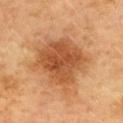Assessment:
This lesion was catalogued during total-body skin photography and was not selected for biopsy.
Acquisition and patient details:
Imaged with cross-polarized lighting. The lesion is located on the upper back. An algorithmic analysis of the crop reported an outline eccentricity of about 0.35 (0 = round, 1 = elongated) and two-axis asymmetry of about 0.2. The analysis additionally found a classifier nevus-likeness of about 90/100 and lesion-presence confidence of about 100/100. A female subject aged around 60. About 6.5 mm across. A 15 mm close-up extracted from a 3D total-body photography capture.